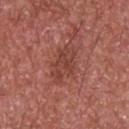  biopsy_status: not biopsied; imaged during a skin examination
  image:
    source: total-body photography crop
    field_of_view_mm: 15
  site: upper back
  lighting: white-light
  patient:
    sex: male
    age_approx: 65
  lesion_size:
    long_diameter_mm_approx: 4.5